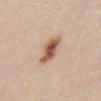Q: Is there a histopathology result?
A: catalogued during a skin exam; not biopsied
Q: Automated lesion metrics?
A: a lesion area of about 7.5 mm² and an outline eccentricity of about 0.85 (0 = round, 1 = elongated); internal color variation of about 5.5 on a 0–10 scale and peripheral color asymmetry of about 1.5; a nevus-likeness score of about 95/100
Q: What is the lesion's diameter?
A: about 4 mm
Q: Where on the body is the lesion?
A: the abdomen
Q: What are the patient's age and sex?
A: female, aged approximately 25
Q: How was the tile lit?
A: white-light
Q: What is the imaging modality?
A: ~15 mm crop, total-body skin-cancer survey